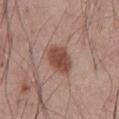Imaged during a routine full-body skin examination; the lesion was not biopsied and no histopathology is available. A roughly 15 mm field-of-view crop from a total-body skin photograph. A male subject aged 58–62. An algorithmic analysis of the crop reported a footprint of about 9.5 mm², a shape eccentricity near 0.7, and two-axis asymmetry of about 0.2. And it measured a lesion–skin lightness drop of about 12 and a lesion-to-skin contrast of about 9 (normalized; higher = more distinct). And it measured a border-irregularity rating of about 2/10, a color-variation rating of about 3.5/10, and a peripheral color-asymmetry measure near 1. On the abdomen. This is a white-light tile.